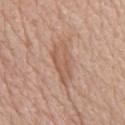Assessment: No biopsy was performed on this lesion — it was imaged during a full skin examination and was not determined to be concerning. Background: Imaged with white-light lighting. Automated image analysis of the tile measured a shape eccentricity near 0.9 and a symmetry-axis asymmetry near 0.3. The analysis additionally found a border-irregularity rating of about 4.5/10 and radial color variation of about 1. The lesion's longest dimension is about 5.5 mm. Located on the chest. This image is a 15 mm lesion crop taken from a total-body photograph. A male subject, aged approximately 75.